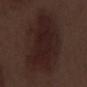| field | value |
|---|---|
| biopsy status | no biopsy performed (imaged during a skin exam) |
| body site | the right lower leg |
| size | ~10.5 mm (longest diameter) |
| illumination | white-light illumination |
| subject | male, aged 68 to 72 |
| automated lesion analysis | a lesion area of about 50 mm², an outline eccentricity of about 0.8 (0 = round, 1 = elongated), and a symmetry-axis asymmetry near 0.2; a mean CIELAB color near L≈20 a*≈16 b*≈16, a lesion–skin lightness drop of about 7, and a lesion-to-skin contrast of about 8.5 (normalized; higher = more distinct) |
| acquisition | ~15 mm tile from a whole-body skin photo |